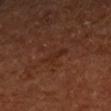The lesion was tiled from a total-body skin photograph and was not biopsied. The patient is a female aged approximately 65. A 15 mm close-up tile from a total-body photography series done for melanoma screening. Automated tile analysis of the lesion measured a border-irregularity index near 4/10 and a within-lesion color-variation index near 0.5/10. The software also gave a classifier nevus-likeness of about 0/100 and a detector confidence of about 100 out of 100 that the crop contains a lesion. Located on the left forearm.